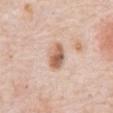notes: imaged on a skin check; not biopsied
lighting: white-light
body site: the abdomen
automated metrics: an outline eccentricity of about 0.75 (0 = round, 1 = elongated) and a shape-asymmetry score of about 0.15 (0 = symmetric); a mean CIELAB color near L≈63 a*≈20 b*≈30, a lesion–skin lightness drop of about 13, and a normalized border contrast of about 8.5; a nevus-likeness score of about 75/100
patient: male, in their 80s
imaging modality: ~15 mm crop, total-body skin-cancer survey
size: ~3.5 mm (longest diameter)